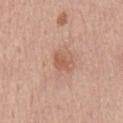{
  "biopsy_status": "not biopsied; imaged during a skin examination",
  "patient": {
    "sex": "male",
    "age_approx": 75
  },
  "automated_metrics": {
    "cielab_L": 57,
    "cielab_a": 23,
    "cielab_b": 31,
    "vs_skin_darker_L": 8.0,
    "vs_skin_contrast_norm": 6.0,
    "color_variation_0_10": 1.0,
    "peripheral_color_asymmetry": 0.5,
    "nevus_likeness_0_100": 0,
    "lesion_detection_confidence_0_100": 100
  },
  "site": "chest",
  "lighting": "white-light",
  "lesion_size": {
    "long_diameter_mm_approx": 2.5
  },
  "image": {
    "source": "total-body photography crop",
    "field_of_view_mm": 15
  }
}